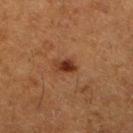Recorded during total-body skin imaging; not selected for excision or biopsy.
A roughly 15 mm field-of-view crop from a total-body skin photograph.
A female subject roughly 50 years of age.
Located on the left lower leg.